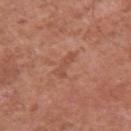{
  "biopsy_status": "not biopsied; imaged during a skin examination",
  "image": {
    "source": "total-body photography crop",
    "field_of_view_mm": 15
  },
  "lighting": "white-light",
  "site": "left upper arm",
  "automated_metrics": {
    "area_mm2_approx": 4.0,
    "eccentricity": 0.9,
    "shape_asymmetry": 0.3,
    "nevus_likeness_0_100": 0,
    "lesion_detection_confidence_0_100": 100
  },
  "patient": {
    "sex": "male",
    "age_approx": 65
  }
}